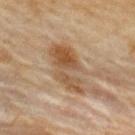Assessment:
Imaged during a routine full-body skin examination; the lesion was not biopsied and no histopathology is available.
Image and clinical context:
A region of skin cropped from a whole-body photographic capture, roughly 15 mm wide. Imaged with cross-polarized lighting. The recorded lesion diameter is about 7 mm. A female subject, about 60 years old. The lesion is on the back.